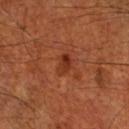Impression:
Part of a total-body skin-imaging series; this lesion was reviewed on a skin check and was not flagged for biopsy.
Acquisition and patient details:
The lesion is on the right thigh. A 15 mm close-up extracted from a 3D total-body photography capture. A male subject aged 68–72.